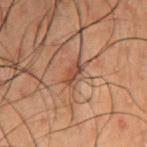Recorded during total-body skin imaging; not selected for excision or biopsy. Automated image analysis of the tile measured a lesion area of about 3 mm² and two-axis asymmetry of about 0.3. It also reported a mean CIELAB color near L≈34 a*≈19 b*≈24, a lesion–skin lightness drop of about 8, and a normalized border contrast of about 7.5. The analysis additionally found an automated nevus-likeness rating near 0 out of 100 and lesion-presence confidence of about 90/100. A region of skin cropped from a whole-body photographic capture, roughly 15 mm wide. From the front of the torso. The patient is a male about 50 years old.